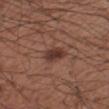Clinical summary:
Cropped from a total-body skin-imaging series; the visible field is about 15 mm. Captured under white-light illumination. The patient is a male approximately 55 years of age. Measured at roughly 3 mm in maximum diameter. On the right forearm.